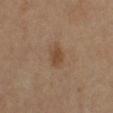Findings:
• workup · catalogued during a skin exam; not biopsied
• automated lesion analysis · a nevus-likeness score of about 45/100 and a lesion-detection confidence of about 100/100
• patient · female, aged around 65
• diameter · ~2.5 mm (longest diameter)
• image · ~15 mm crop, total-body skin-cancer survey
• anatomic site · the left leg
• illumination · cross-polarized